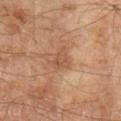From the left upper arm. A male patient, in their mid- to late 40s. Approximately 3 mm at its widest. A close-up tile cropped from a whole-body skin photograph, about 15 mm across. Imaged with cross-polarized lighting.